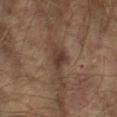Q: Was a biopsy performed?
A: catalogued during a skin exam; not biopsied
Q: Patient demographics?
A: male, aged 63 to 67
Q: What is the anatomic site?
A: the left forearm
Q: Automated lesion metrics?
A: a footprint of about 5.5 mm², an eccentricity of roughly 0.85, and a symmetry-axis asymmetry near 0.4; a mean CIELAB color near L≈35 a*≈17 b*≈22 and a normalized lesion–skin contrast near 8.5; a color-variation rating of about 3/10 and a peripheral color-asymmetry measure near 1; an automated nevus-likeness rating near 55 out of 100
Q: How large is the lesion?
A: about 4 mm
Q: How was the tile lit?
A: cross-polarized illumination
Q: How was this image acquired?
A: total-body-photography crop, ~15 mm field of view